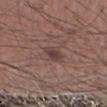A region of skin cropped from a whole-body photographic capture, roughly 15 mm wide. The subject is a male aged 63–67. The lesion is on the right forearm.